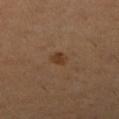Notes:
- follow-up · catalogued during a skin exam; not biopsied
- image · ~15 mm crop, total-body skin-cancer survey
- subject · female, aged 28–32
- body site · the right lower leg